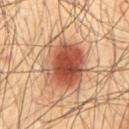biopsy status: catalogued during a skin exam; not biopsied | tile lighting: cross-polarized illumination | subject: male, in their mid- to late 60s | lesion size: ≈7 mm | acquisition: ~15 mm tile from a whole-body skin photo | body site: the back.